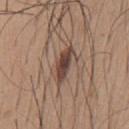anatomic site: the chest | illumination: white-light illumination | TBP lesion metrics: an area of roughly 6.5 mm², an outline eccentricity of about 0.95 (0 = round, 1 = elongated), and a symmetry-axis asymmetry near 0.25; a border-irregularity index near 3.5/10 and radial color variation of about 1 | diameter: ≈4.5 mm | patient: male, approximately 65 years of age | image source: ~15 mm crop, total-body skin-cancer survey.